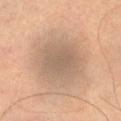follow-up — total-body-photography surveillance lesion; no biopsy
patient — male, aged 43 to 47
image — ~15 mm tile from a whole-body skin photo
diameter — about 5 mm
body site — the right upper arm
tile lighting — cross-polarized
image-analysis metrics — a shape eccentricity near 0.45 and two-axis asymmetry of about 0.1; a border-irregularity index near 1.5/10, a color-variation rating of about 2.5/10, and radial color variation of about 0.5